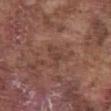{"biopsy_status": "not biopsied; imaged during a skin examination", "site": "abdomen", "lighting": "white-light", "image": {"source": "total-body photography crop", "field_of_view_mm": 15}, "lesion_size": {"long_diameter_mm_approx": 3.0}, "patient": {"sex": "male", "age_approx": 75}}